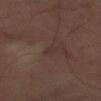Recorded during total-body skin imaging; not selected for excision or biopsy.
About 1 mm across.
A lesion tile, about 15 mm wide, cut from a 3D total-body photograph.
The lesion is on the left upper arm.
An algorithmic analysis of the crop reported a nevus-likeness score of about 0/100 and a lesion-detection confidence of about 80/100.
A male patient aged approximately 65.
The tile uses cross-polarized illumination.